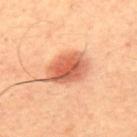{"biopsy_status": "not biopsied; imaged during a skin examination", "patient": {"sex": "male", "age_approx": 65}, "site": "upper back", "lighting": "cross-polarized", "automated_metrics": {"area_mm2_approx": 14.0, "eccentricity": 0.75, "shape_asymmetry": 0.2, "cielab_L": 61, "cielab_a": 29, "cielab_b": 36, "vs_skin_darker_L": 15.0}, "image": {"source": "total-body photography crop", "field_of_view_mm": 15}}